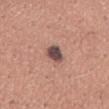Clinical impression: Captured during whole-body skin photography for melanoma surveillance; the lesion was not biopsied. Image and clinical context: The patient is a male aged 43–47. Located on the mid back. The lesion-visualizer software estimated an area of roughly 5 mm² and a shape eccentricity near 0.5. And it measured a mean CIELAB color near L≈47 a*≈18 b*≈20, roughly 16 lightness units darker than nearby skin, and a lesion-to-skin contrast of about 12 (normalized; higher = more distinct). Imaged with white-light lighting. A 15 mm crop from a total-body photograph taken for skin-cancer surveillance. About 2.5 mm across.